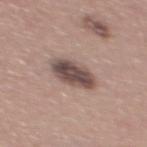| key | value |
|---|---|
| notes | total-body-photography surveillance lesion; no biopsy |
| lesion size | ~4.5 mm (longest diameter) |
| body site | the mid back |
| lighting | white-light |
| patient | male, aged around 40 |
| automated metrics | border irregularity of about 1.5 on a 0–10 scale, internal color variation of about 4.5 on a 0–10 scale, and radial color variation of about 1.5; a classifier nevus-likeness of about 50/100 and a detector confidence of about 100 out of 100 that the crop contains a lesion |
| acquisition | 15 mm crop, total-body photography |